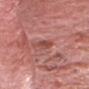Context: This image is a 15 mm lesion crop taken from a total-body photograph. Automated image analysis of the tile measured an area of roughly 3.5 mm², a shape eccentricity near 0.85, and a symmetry-axis asymmetry near 0.25. The analysis additionally found an average lesion color of about L≈49 a*≈28 b*≈27 (CIELAB), about 9 CIELAB-L* units darker than the surrounding skin, and a normalized border contrast of about 6.5. It also reported a within-lesion color-variation index near 1.5/10 and peripheral color asymmetry of about 0.5. The lesion is located on the head or neck. A male patient in their mid-70s.